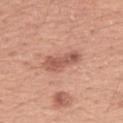Q: Was a biopsy performed?
A: no biopsy performed (imaged during a skin exam)
Q: What is the anatomic site?
A: the left forearm
Q: Patient demographics?
A: female, in their mid-40s
Q: What kind of image is this?
A: ~15 mm tile from a whole-body skin photo
Q: Illumination type?
A: white-light illumination
Q: How large is the lesion?
A: about 4.5 mm